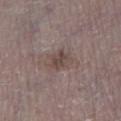biopsy status: imaged on a skin check; not biopsied | anatomic site: the leg | subject: male, aged approximately 70 | acquisition: 15 mm crop, total-body photography | lighting: white-light illumination | size: ~3 mm (longest diameter) | automated metrics: a lesion color around L≈45 a*≈14 b*≈19 in CIELAB, about 8 CIELAB-L* units darker than the surrounding skin, and a normalized border contrast of about 6.5; a border-irregularity index near 2.5/10 and internal color variation of about 4 on a 0–10 scale.